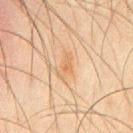follow-up: imaged on a skin check; not biopsied | imaging modality: ~15 mm tile from a whole-body skin photo | location: the chest | subject: male, aged around 45.